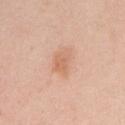The lesion was photographed on a routine skin check and not biopsied; there is no pathology result. A 15 mm crop from a total-body photograph taken for skin-cancer surveillance. A female subject, approximately 25 years of age. The lesion is located on the left upper arm.